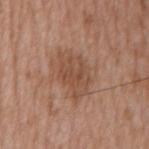Clinical impression:
The lesion was photographed on a routine skin check and not biopsied; there is no pathology result.
Background:
Automated image analysis of the tile measured a lesion color around L≈49 a*≈20 b*≈30 in CIELAB, roughly 8 lightness units darker than nearby skin, and a lesion-to-skin contrast of about 6 (normalized; higher = more distinct). And it measured a border-irregularity rating of about 3.5/10 and a peripheral color-asymmetry measure near 1. A lesion tile, about 15 mm wide, cut from a 3D total-body photograph. A male patient aged 73 to 77. On the mid back.